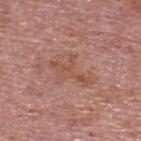The lesion was photographed on a routine skin check and not biopsied; there is no pathology result. The recorded lesion diameter is about 5 mm. The lesion is on the upper back. A male patient, aged around 75. This image is a 15 mm lesion crop taken from a total-body photograph. The total-body-photography lesion software estimated an area of roughly 9.5 mm², a shape eccentricity near 0.85, and a shape-asymmetry score of about 0.6 (0 = symmetric). The software also gave an automated nevus-likeness rating near 0 out of 100 and a lesion-detection confidence of about 100/100.